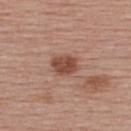Clinical impression: This lesion was catalogued during total-body skin photography and was not selected for biopsy. Context: The patient is a female roughly 55 years of age. A lesion tile, about 15 mm wide, cut from a 3D total-body photograph. Longest diameter approximately 3.5 mm. This is a white-light tile. From the back.